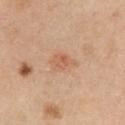Assessment: Part of a total-body skin-imaging series; this lesion was reviewed on a skin check and was not flagged for biopsy. Context: A female subject about 45 years old. The lesion is on the chest. Captured under white-light illumination. This image is a 15 mm lesion crop taken from a total-body photograph.